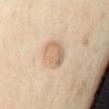Impression: The lesion was photographed on a routine skin check and not biopsied; there is no pathology result. Acquisition and patient details: A female patient, aged 38–42. Longest diameter approximately 3.5 mm. This image is a 15 mm lesion crop taken from a total-body photograph. Automated image analysis of the tile measured a footprint of about 10 mm², an outline eccentricity of about 0.4 (0 = round, 1 = elongated), and a shape-asymmetry score of about 0.2 (0 = symmetric). The software also gave a lesion color around L≈68 a*≈15 b*≈33 in CIELAB, a lesion–skin lightness drop of about 9, and a lesion-to-skin contrast of about 6 (normalized; higher = more distinct). It also reported a detector confidence of about 100 out of 100 that the crop contains a lesion. The tile uses cross-polarized illumination. The lesion is located on the chest.